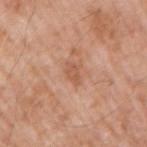The lesion was tiled from a total-body skin photograph and was not biopsied.